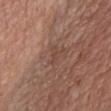The lesion was photographed on a routine skin check and not biopsied; there is no pathology result. A region of skin cropped from a whole-body photographic capture, roughly 15 mm wide. Imaged with white-light lighting. The lesion's longest dimension is about 2.5 mm. A male subject aged approximately 80. Located on the front of the torso.